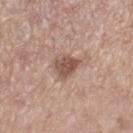The lesion was tiled from a total-body skin photograph and was not biopsied.
This is a white-light tile.
A female patient, aged around 55.
A region of skin cropped from a whole-body photographic capture, roughly 15 mm wide.
The lesion is located on the left thigh.
An algorithmic analysis of the crop reported a lesion area of about 6.5 mm² and two-axis asymmetry of about 0.4.